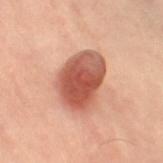<lesion>
  <biopsy_status>not biopsied; imaged during a skin examination</biopsy_status>
  <lesion_size>
    <long_diameter_mm_approx>6.0</long_diameter_mm_approx>
  </lesion_size>
  <patient>
    <sex>female</sex>
    <age_approx>60</age_approx>
  </patient>
  <site>left leg</site>
  <image>
    <source>total-body photography crop</source>
    <field_of_view_mm>15</field_of_view_mm>
  </image>
</lesion>